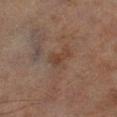Recorded during total-body skin imaging; not selected for excision or biopsy. A 15 mm crop from a total-body photograph taken for skin-cancer surveillance. Approximately 2.5 mm at its widest. The lesion is located on the right lower leg. The tile uses cross-polarized illumination. A male subject, approximately 70 years of age. An algorithmic analysis of the crop reported a lesion area of about 3.5 mm², a shape eccentricity near 0.85, and a symmetry-axis asymmetry near 0.5. The software also gave a mean CIELAB color near L≈32 a*≈15 b*≈22, a lesion–skin lightness drop of about 6, and a lesion-to-skin contrast of about 6 (normalized; higher = more distinct). And it measured a nevus-likeness score of about 0/100 and a detector confidence of about 100 out of 100 that the crop contains a lesion.